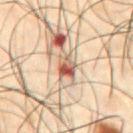Assessment: Captured during whole-body skin photography for melanoma surveillance; the lesion was not biopsied. Context: The patient is a male aged 48 to 52. A 15 mm close-up tile from a total-body photography series done for melanoma screening. Located on the front of the torso. Captured under cross-polarized illumination.